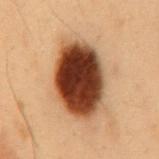The subject is a male aged 53 to 57. On the mid back. A roughly 15 mm field-of-view crop from a total-body skin photograph.